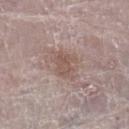Q: Was a biopsy performed?
A: catalogued during a skin exam; not biopsied
Q: How was the tile lit?
A: white-light
Q: What is the imaging modality?
A: total-body-photography crop, ~15 mm field of view
Q: What is the anatomic site?
A: the left lower leg
Q: Automated lesion metrics?
A: a symmetry-axis asymmetry near 0.3; a mean CIELAB color near L≈54 a*≈17 b*≈22 and a normalized lesion–skin contrast near 6; a border-irregularity index near 4/10, internal color variation of about 2 on a 0–10 scale, and peripheral color asymmetry of about 1; a nevus-likeness score of about 0/100
Q: How large is the lesion?
A: about 3.5 mm
Q: Patient demographics?
A: female, roughly 65 years of age